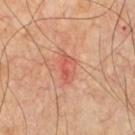Captured under cross-polarized illumination. On the chest. A region of skin cropped from a whole-body photographic capture, roughly 15 mm wide. A male subject, aged 58–62.